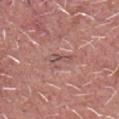notes: imaged on a skin check; not biopsied | image-analysis metrics: a lesion area of about 3.5 mm², a shape eccentricity near 0.85, and a shape-asymmetry score of about 0.5 (0 = symmetric); peripheral color asymmetry of about 0; a classifier nevus-likeness of about 0/100 and a detector confidence of about 90 out of 100 that the crop contains a lesion | patient: male, in their 60s | imaging modality: ~15 mm tile from a whole-body skin photo | site: the head or neck | illumination: white-light illumination | lesion diameter: ≈3 mm.